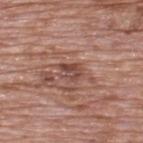No biopsy was performed on this lesion — it was imaged during a full skin examination and was not determined to be concerning.
A lesion tile, about 15 mm wide, cut from a 3D total-body photograph.
Captured under white-light illumination.
The lesion's longest dimension is about 2.5 mm.
An algorithmic analysis of the crop reported a lesion area of about 4.5 mm², a shape eccentricity near 0.6, and two-axis asymmetry of about 0.25. It also reported an average lesion color of about L≈46 a*≈22 b*≈25 (CIELAB) and a lesion-to-skin contrast of about 7 (normalized; higher = more distinct).
From the back.
A male subject, approximately 70 years of age.